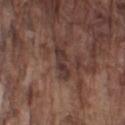  image:
    source: total-body photography crop
    field_of_view_mm: 15
  site: left upper arm
  patient:
    sex: male
    age_approx: 75